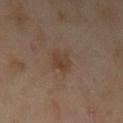notes = no biopsy performed (imaged during a skin exam)
image source = total-body-photography crop, ~15 mm field of view
lighting = cross-polarized illumination
subject = female, approximately 40 years of age
site = the left upper arm
diameter = about 2.5 mm